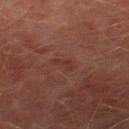• imaging modality — total-body-photography crop, ~15 mm field of view
• automated lesion analysis — an outline eccentricity of about 0.95 (0 = round, 1 = elongated) and two-axis asymmetry of about 0.55; border irregularity of about 6 on a 0–10 scale, a color-variation rating of about 0/10, and radial color variation of about 0
• anatomic site — the right thigh
• patient — male, approximately 75 years of age
• tile lighting — cross-polarized illumination
• diameter — about 3 mm